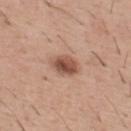Q: Was a biopsy performed?
A: total-body-photography surveillance lesion; no biopsy
Q: How large is the lesion?
A: ≈3.5 mm
Q: Where on the body is the lesion?
A: the mid back
Q: Who is the patient?
A: male, aged 38–42
Q: How was this image acquired?
A: ~15 mm crop, total-body skin-cancer survey